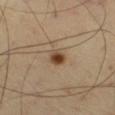<record>
<biopsy_status>not biopsied; imaged during a skin examination</biopsy_status>
<image>
  <source>total-body photography crop</source>
  <field_of_view_mm>15</field_of_view_mm>
</image>
<site>right thigh</site>
<patient>
  <sex>male</sex>
  <age_approx>55</age_approx>
</patient>
</record>